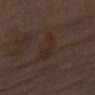{"biopsy_status": "not biopsied; imaged during a skin examination", "lighting": "cross-polarized", "patient": {"sex": "female", "age_approx": 65}, "site": "left upper arm", "image": {"source": "total-body photography crop", "field_of_view_mm": 15}}